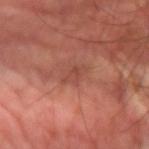{
  "site": "arm",
  "patient": {
    "sex": "male",
    "age_approx": 60
  },
  "automated_metrics": {
    "border_irregularity_0_10": 4.0,
    "color_variation_0_10": 1.5,
    "peripheral_color_asymmetry": 0.5
  },
  "image": {
    "source": "total-body photography crop",
    "field_of_view_mm": 15
  },
  "lesion_size": {
    "long_diameter_mm_approx": 3.0
  }
}